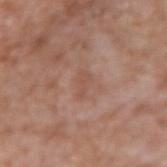Assessment: Captured during whole-body skin photography for melanoma surveillance; the lesion was not biopsied. Background: The lesion is on the arm. This is a white-light tile. The lesion-visualizer software estimated an area of roughly 3.5 mm² and an eccentricity of roughly 0.85. The software also gave roughly 6 lightness units darker than nearby skin and a lesion-to-skin contrast of about 4.5 (normalized; higher = more distinct). About 3 mm across. The subject is a male in their mid-70s. Cropped from a total-body skin-imaging series; the visible field is about 15 mm.